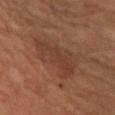Case summary:
• notes: no biopsy performed (imaged during a skin exam)
• image: total-body-photography crop, ~15 mm field of view
• location: the right forearm
• subject: male, about 55 years old